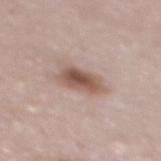Q: Was a biopsy performed?
A: no biopsy performed (imaged during a skin exam)
Q: How was the tile lit?
A: white-light
Q: Automated lesion metrics?
A: a lesion area of about 9 mm², a shape eccentricity near 0.85, and two-axis asymmetry of about 0.2; border irregularity of about 2.5 on a 0–10 scale and internal color variation of about 5 on a 0–10 scale; an automated nevus-likeness rating near 95 out of 100 and a detector confidence of about 100 out of 100 that the crop contains a lesion
Q: How was this image acquired?
A: ~15 mm crop, total-body skin-cancer survey
Q: Patient demographics?
A: female, aged 48–52
Q: Where on the body is the lesion?
A: the mid back
Q: How large is the lesion?
A: about 4.5 mm